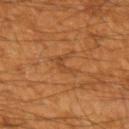The lesion was tiled from a total-body skin photograph and was not biopsied.
This is a cross-polarized tile.
The patient is a male aged 58–62.
The lesion's longest dimension is about 3 mm.
From the left upper arm.
Cropped from a whole-body photographic skin survey; the tile spans about 15 mm.
The total-body-photography lesion software estimated a lesion area of about 3.5 mm², an outline eccentricity of about 0.75 (0 = round, 1 = elongated), and two-axis asymmetry of about 0.6. The software also gave an average lesion color of about L≈41 a*≈22 b*≈36 (CIELAB) and roughly 5 lightness units darker than nearby skin. And it measured an automated nevus-likeness rating near 0 out of 100 and a detector confidence of about 95 out of 100 that the crop contains a lesion.